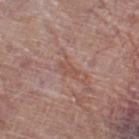Captured during whole-body skin photography for melanoma surveillance; the lesion was not biopsied.
A female patient, aged 68 to 72.
This is a white-light tile.
The recorded lesion diameter is about 2.5 mm.
The lesion is located on the right thigh.
Cropped from a total-body skin-imaging series; the visible field is about 15 mm.
Automated image analysis of the tile measured a lesion color around L≈51 a*≈21 b*≈26 in CIELAB, roughly 6 lightness units darker than nearby skin, and a normalized border contrast of about 5.5. And it measured an automated nevus-likeness rating near 0 out of 100 and lesion-presence confidence of about 95/100.